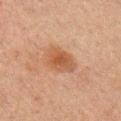Image and clinical context:
A 15 mm close-up tile from a total-body photography series done for melanoma screening. Longest diameter approximately 4 mm. The subject is a male aged 48–52. The tile uses cross-polarized illumination. The lesion is located on the right upper arm.